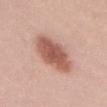Q: Was a biopsy performed?
A: catalogued during a skin exam; not biopsied
Q: What is the lesion's diameter?
A: ≈6.5 mm
Q: Automated lesion metrics?
A: about 15 CIELAB-L* units darker than the surrounding skin and a normalized border contrast of about 9.5; a border-irregularity rating of about 2/10, internal color variation of about 3.5 on a 0–10 scale, and a peripheral color-asymmetry measure near 1
Q: What are the patient's age and sex?
A: female, approximately 25 years of age
Q: Lesion location?
A: the front of the torso
Q: Illumination type?
A: white-light
Q: What is the imaging modality?
A: total-body-photography crop, ~15 mm field of view